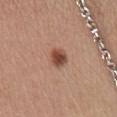Impression: No biopsy was performed on this lesion — it was imaged during a full skin examination and was not determined to be concerning. Clinical summary: The lesion is located on the chest. Cropped from a total-body skin-imaging series; the visible field is about 15 mm. About 2.5 mm across. A female subject, in their mid- to late 40s.